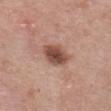Imaged during a routine full-body skin examination; the lesion was not biopsied and no histopathology is available.
Located on the front of the torso.
The tile uses white-light illumination.
The recorded lesion diameter is about 4 mm.
The patient is a female aged around 65.
A roughly 15 mm field-of-view crop from a total-body skin photograph.